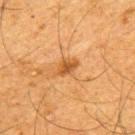workup = no biopsy performed (imaged during a skin exam) | lighting = cross-polarized | lesion size = ~3 mm (longest diameter) | site = the upper back | image = ~15 mm tile from a whole-body skin photo | patient = male, aged 63–67.